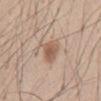Case summary:
* biopsy status — total-body-photography surveillance lesion; no biopsy
* acquisition — ~15 mm tile from a whole-body skin photo
* body site — the abdomen
* subject — male, aged 43 to 47